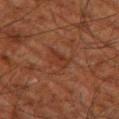A male patient aged approximately 80. On the leg. A 15 mm close-up extracted from a 3D total-body photography capture.